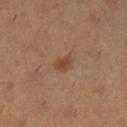Case summary:
– follow-up — catalogued during a skin exam; not biopsied
– subject — female, aged 28 to 32
– location — the left lower leg
– TBP lesion metrics — a shape eccentricity near 0.75 and a shape-asymmetry score of about 0.25 (0 = symmetric); a lesion–skin lightness drop of about 8 and a normalized lesion–skin contrast near 7; a detector confidence of about 100 out of 100 that the crop contains a lesion
– lesion size — about 2.5 mm
– imaging modality — 15 mm crop, total-body photography
– illumination — cross-polarized illumination